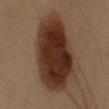• biopsy status · total-body-photography surveillance lesion; no biopsy
• lesion diameter · about 8 mm
• body site · the left upper arm
• lighting · cross-polarized
• subject · male, aged around 55
• image · 15 mm crop, total-body photography